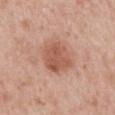<case>
<biopsy_status>not biopsied; imaged during a skin examination</biopsy_status>
<lighting>white-light</lighting>
<image>
  <source>total-body photography crop</source>
  <field_of_view_mm>15</field_of_view_mm>
</image>
<patient>
  <sex>male</sex>
  <age_approx>65</age_approx>
</patient>
<site>mid back</site>
<automated_metrics>
  <eccentricity>0.4</eccentricity>
  <border_irregularity_0_10>1.5</border_irregularity_0_10>
</automated_metrics>
</case>